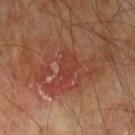Assessment:
Imaged during a routine full-body skin examination; the lesion was not biopsied and no histopathology is available.